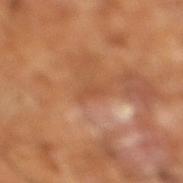Case summary:
– follow-up: no biopsy performed (imaged during a skin exam)